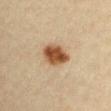The lesion was photographed on a routine skin check and not biopsied; there is no pathology result.